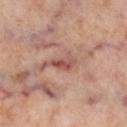Clinical impression:
The lesion was tiled from a total-body skin photograph and was not biopsied.
Image and clinical context:
This image is a 15 mm lesion crop taken from a total-body photograph. The lesion-visualizer software estimated a footprint of about 3.5 mm², an eccentricity of roughly 0.9, and a shape-asymmetry score of about 0.45 (0 = symmetric). The software also gave a lesion color around L≈52 a*≈25 b*≈25 in CIELAB, about 11 CIELAB-L* units darker than the surrounding skin, and a normalized border contrast of about 7.5. The analysis additionally found a nevus-likeness score of about 0/100 and lesion-presence confidence of about 75/100. Located on the right lower leg. The patient is a female aged around 55. Captured under cross-polarized illumination.